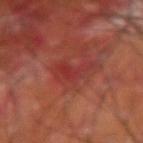The lesion was tiled from a total-body skin photograph and was not biopsied. A roughly 15 mm field-of-view crop from a total-body skin photograph. The tile uses cross-polarized illumination. Longest diameter approximately 4 mm. The subject is a male approximately 60 years of age. The total-body-photography lesion software estimated a mean CIELAB color near L≈37 a*≈33 b*≈27, a lesion–skin lightness drop of about 6, and a normalized border contrast of about 5. And it measured a nevus-likeness score of about 0/100 and lesion-presence confidence of about 95/100. On the right upper arm.